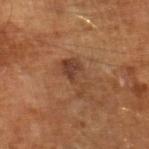workup: catalogued during a skin exam; not biopsied
body site: the left leg
lesion diameter: ~4.5 mm (longest diameter)
image source: ~15 mm crop, total-body skin-cancer survey
patient: male, approximately 65 years of age
illumination: cross-polarized